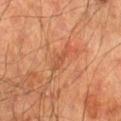Context: A male subject, in their mid- to late 60s. The tile uses cross-polarized illumination. Approximately 2.5 mm at its widest. Located on the right lower leg. Cropped from a total-body skin-imaging series; the visible field is about 15 mm.